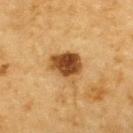Impression: Part of a total-body skin-imaging series; this lesion was reviewed on a skin check and was not flagged for biopsy. Context: About 4.5 mm across. The lesion-visualizer software estimated a lesion color around L≈42 a*≈19 b*≈36 in CIELAB and a lesion-to-skin contrast of about 11.5 (normalized; higher = more distinct). The patient is a male roughly 85 years of age or older. Located on the upper back. A close-up tile cropped from a whole-body skin photograph, about 15 mm across. The tile uses cross-polarized illumination.